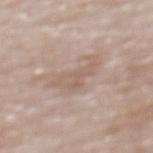workup — imaged on a skin check; not biopsied
location — the upper back
subject — male, in their mid- to late 80s or older
acquisition — total-body-photography crop, ~15 mm field of view
size — about 5 mm